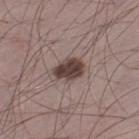Part of a total-body skin-imaging series; this lesion was reviewed on a skin check and was not flagged for biopsy. The lesion's longest dimension is about 4 mm. A male subject, in their mid- to late 30s. Located on the left thigh. Automated tile analysis of the lesion measured a classifier nevus-likeness of about 75/100 and lesion-presence confidence of about 100/100. A roughly 15 mm field-of-view crop from a total-body skin photograph.